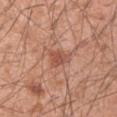follow-up = catalogued during a skin exam; not biopsied
patient = male, aged 58 to 62
body site = the left upper arm
illumination = white-light
image source = total-body-photography crop, ~15 mm field of view
diameter = ≈2.5 mm